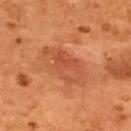workup: imaged on a skin check; not biopsied
subject: male, in their mid- to late 50s
image source: total-body-photography crop, ~15 mm field of view
automated lesion analysis: an area of roughly 11 mm²; a lesion color around L≈47 a*≈29 b*≈36 in CIELAB, roughly 7 lightness units darker than nearby skin, and a lesion-to-skin contrast of about 5.5 (normalized; higher = more distinct); a lesion-detection confidence of about 100/100
illumination: cross-polarized illumination
anatomic site: the upper back
size: ≈5.5 mm